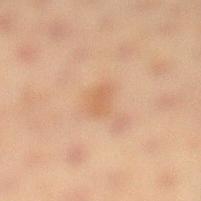Part of a total-body skin-imaging series; this lesion was reviewed on a skin check and was not flagged for biopsy. This is a cross-polarized tile. A roughly 15 mm field-of-view crop from a total-body skin photograph. On the left lower leg. Longest diameter approximately 2.5 mm. A female subject aged 18 to 22. Automated image analysis of the tile measured a shape eccentricity near 0.75. The analysis additionally found a mean CIELAB color near L≈54 a*≈19 b*≈32, a lesion–skin lightness drop of about 6, and a lesion-to-skin contrast of about 5 (normalized; higher = more distinct). The analysis additionally found a border-irregularity index near 2.5/10, a within-lesion color-variation index near 1.5/10, and radial color variation of about 0.5. The software also gave a nevus-likeness score of about 15/100 and a lesion-detection confidence of about 100/100.